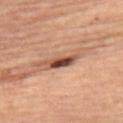| key | value |
|---|---|
| biopsy status | no biopsy performed (imaged during a skin exam) |
| automated metrics | an average lesion color of about L≈46 a*≈23 b*≈30 (CIELAB) and a normalized lesion–skin contrast near 12; a border-irregularity index near 4.5/10, a color-variation rating of about 5/10, and radial color variation of about 1 |
| patient | female, approximately 70 years of age |
| illumination | cross-polarized |
| image source | ~15 mm tile from a whole-body skin photo |
| size | ~4.5 mm (longest diameter) |
| site | the right thigh |